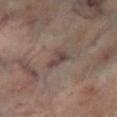Q: Was this lesion biopsied?
A: total-body-photography surveillance lesion; no biopsy
Q: What lighting was used for the tile?
A: cross-polarized illumination
Q: Patient demographics?
A: male, approximately 70 years of age
Q: What did automated image analysis measure?
A: a lesion color around L≈40 a*≈15 b*≈17 in CIELAB, roughly 9 lightness units darker than nearby skin, and a lesion-to-skin contrast of about 8 (normalized; higher = more distinct); an automated nevus-likeness rating near 0 out of 100 and a detector confidence of about 80 out of 100 that the crop contains a lesion
Q: Lesion location?
A: the left lower leg
Q: How was this image acquired?
A: total-body-photography crop, ~15 mm field of view
Q: What is the lesion's diameter?
A: about 3 mm